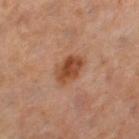follow-up: total-body-photography surveillance lesion; no biopsy | body site: the left thigh | size: ≈3.5 mm | image: ~15 mm tile from a whole-body skin photo | illumination: cross-polarized | patient: female, roughly 55 years of age | image-analysis metrics: a lesion color around L≈43 a*≈22 b*≈32 in CIELAB; a border-irregularity index near 2/10, a color-variation rating of about 4/10, and radial color variation of about 1.5; an automated nevus-likeness rating near 90 out of 100.